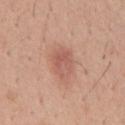{
  "biopsy_status": "not biopsied; imaged during a skin examination",
  "patient": {
    "sex": "male",
    "age_approx": 40
  },
  "image": {
    "source": "total-body photography crop",
    "field_of_view_mm": 15
  },
  "site": "chest"
}